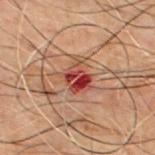An algorithmic analysis of the crop reported a lesion area of about 7.5 mm², a shape eccentricity near 0.6, and a symmetry-axis asymmetry near 0.3. And it measured a mean CIELAB color near L≈34 a*≈25 b*≈24 and roughly 11 lightness units darker than nearby skin. The analysis additionally found a classifier nevus-likeness of about 0/100 and a detector confidence of about 100 out of 100 that the crop contains a lesion. Located on the chest. The subject is a male approximately 50 years of age. Approximately 3.5 mm at its widest. A region of skin cropped from a whole-body photographic capture, roughly 15 mm wide. The tile uses cross-polarized illumination.